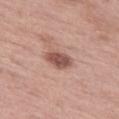Impression:
This lesion was catalogued during total-body skin photography and was not selected for biopsy.
Image and clinical context:
A roughly 15 mm field-of-view crop from a total-body skin photograph. A female patient, in their 70s. Located on the leg.